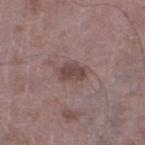{"biopsy_status": "not biopsied; imaged during a skin examination", "site": "left thigh", "image": {"source": "total-body photography crop", "field_of_view_mm": 15}, "patient": {"sex": "male", "age_approx": 50}}